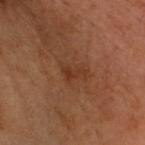The lesion was tiled from a total-body skin photograph and was not biopsied. Measured at roughly 2.5 mm in maximum diameter. A roughly 15 mm field-of-view crop from a total-body skin photograph. The patient is a male in their mid-50s. Imaged with cross-polarized lighting. From the head or neck. The lesion-visualizer software estimated a color-variation rating of about 0.5/10 and radial color variation of about 0. The analysis additionally found a nevus-likeness score of about 5/100 and a detector confidence of about 100 out of 100 that the crop contains a lesion.